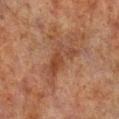Q: Was this lesion biopsied?
A: no biopsy performed (imaged during a skin exam)
Q: How was this image acquired?
A: ~15 mm tile from a whole-body skin photo
Q: Where on the body is the lesion?
A: the right lower leg
Q: Who is the patient?
A: male, aged 68 to 72
Q: What lighting was used for the tile?
A: cross-polarized
Q: How large is the lesion?
A: ≈5 mm